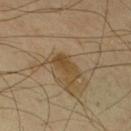Q: Was this lesion biopsied?
A: catalogued during a skin exam; not biopsied
Q: What is the anatomic site?
A: the leg
Q: Who is the patient?
A: male, aged approximately 65
Q: How was this image acquired?
A: ~15 mm crop, total-body skin-cancer survey
Q: What lighting was used for the tile?
A: cross-polarized